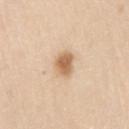follow-up = total-body-photography surveillance lesion; no biopsy
subject = male, roughly 60 years of age
diameter = about 2.5 mm
tile lighting = white-light illumination
anatomic site = the right upper arm
image source = total-body-photography crop, ~15 mm field of view